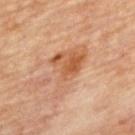Assessment: Recorded during total-body skin imaging; not selected for excision or biopsy. Image and clinical context: Automated tile analysis of the lesion measured an average lesion color of about L≈57 a*≈23 b*≈35 (CIELAB) and a normalized lesion–skin contrast near 7. And it measured a border-irregularity index near 6/10, internal color variation of about 6 on a 0–10 scale, and radial color variation of about 2. The analysis additionally found a classifier nevus-likeness of about 0/100 and lesion-presence confidence of about 100/100. The tile uses cross-polarized illumination. Cropped from a whole-body photographic skin survey; the tile spans about 15 mm. From the upper back. A male subject, approximately 85 years of age or older. The recorded lesion diameter is about 7 mm.